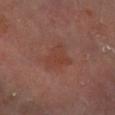– workup — total-body-photography surveillance lesion; no biopsy
– subject — male, in their mid- to late 60s
– body site — the right lower leg
– image source — total-body-photography crop, ~15 mm field of view